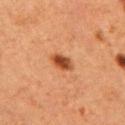The lesion was tiled from a total-body skin photograph and was not biopsied.
A 15 mm close-up tile from a total-body photography series done for melanoma screening.
Captured under cross-polarized illumination.
A female patient aged 38–42.
From the right upper arm.
Approximately 3 mm at its widest.